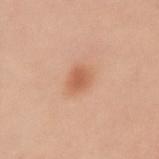Assessment: The lesion was photographed on a routine skin check and not biopsied; there is no pathology result. Clinical summary: The lesion is on the right upper arm. Automated image analysis of the tile measured an average lesion color of about L≈60 a*≈25 b*≈35 (CIELAB), a lesion–skin lightness drop of about 10, and a normalized lesion–skin contrast near 6.5. The analysis additionally found a border-irregularity rating of about 1.5/10 and a peripheral color-asymmetry measure near 1. And it measured a classifier nevus-likeness of about 95/100 and lesion-presence confidence of about 100/100. This image is a 15 mm lesion crop taken from a total-body photograph. The lesion's longest dimension is about 2.5 mm. A female subject in their 40s.